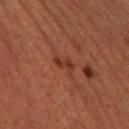workup = catalogued during a skin exam; not biopsied
illumination = cross-polarized
subject = female, aged around 40
site = the leg
image = 15 mm crop, total-body photography
lesion diameter = ≈2.5 mm